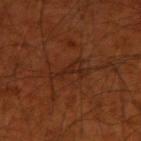• anatomic site — the right upper arm
• imaging modality — total-body-photography crop, ~15 mm field of view
• image-analysis metrics — an area of roughly 5.5 mm² and an outline eccentricity of about 0.9 (0 = round, 1 = elongated)
• patient — male, aged around 70
• illumination — cross-polarized illumination